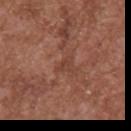Impression:
This lesion was catalogued during total-body skin photography and was not selected for biopsy.
Image and clinical context:
The tile uses white-light illumination. The lesion is on the upper back. A male patient aged around 45. This image is a 15 mm lesion crop taken from a total-body photograph.